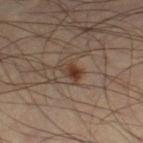notes: catalogued during a skin exam; not biopsied
anatomic site: the left lower leg
image: ~15 mm crop, total-body skin-cancer survey
illumination: cross-polarized
subject: male, in their mid-40s